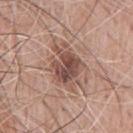Recorded during total-body skin imaging; not selected for excision or biopsy.
A male subject, aged 58–62.
A lesion tile, about 15 mm wide, cut from a 3D total-body photograph.
Approximately 5.5 mm at its widest.
The lesion is on the chest.
Captured under white-light illumination.
An algorithmic analysis of the crop reported a footprint of about 14 mm² and a shape eccentricity near 0.7.